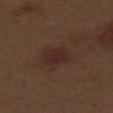Assessment: Part of a total-body skin-imaging series; this lesion was reviewed on a skin check and was not flagged for biopsy. Background: A male patient, aged 68 to 72. The lesion-visualizer software estimated an outline eccentricity of about 0.75 (0 = round, 1 = elongated). It also reported an average lesion color of about L≈26 a*≈18 b*≈21 (CIELAB), roughly 5 lightness units darker than nearby skin, and a normalized border contrast of about 6. The lesion is on the right thigh. This image is a 15 mm lesion crop taken from a total-body photograph.